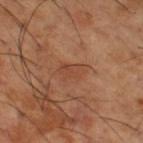follow-up — imaged on a skin check; not biopsied
automated lesion analysis — an outline eccentricity of about 0.85 (0 = round, 1 = elongated) and two-axis asymmetry of about 0.3; a classifier nevus-likeness of about 0/100 and a lesion-detection confidence of about 100/100
size — about 3.5 mm
patient — male, aged 63–67
tile lighting — cross-polarized
location — the right thigh
image source — ~15 mm tile from a whole-body skin photo